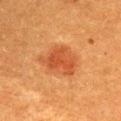Imaged during a routine full-body skin examination; the lesion was not biopsied and no histopathology is available. This is a cross-polarized tile. The lesion's longest dimension is about 5 mm. A female patient, aged 53–57. From the back. A close-up tile cropped from a whole-body skin photograph, about 15 mm across.